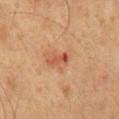Part of a total-body skin-imaging series; this lesion was reviewed on a skin check and was not flagged for biopsy.